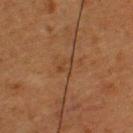patient — male, aged approximately 50
image — total-body-photography crop, ~15 mm field of view
location — the upper back
automated metrics — an eccentricity of roughly 0.7; an average lesion color of about L≈32 a*≈16 b*≈28 (CIELAB), about 5 CIELAB-L* units darker than the surrounding skin, and a lesion-to-skin contrast of about 4.5 (normalized; higher = more distinct); a border-irregularity index near 4.5/10 and radial color variation of about 0
lesion diameter — ~2.5 mm (longest diameter)
tile lighting — cross-polarized